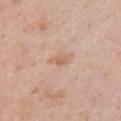Q: Is there a histopathology result?
A: total-body-photography surveillance lesion; no biopsy
Q: Automated lesion metrics?
A: a lesion area of about 3 mm², an eccentricity of roughly 0.85, and a symmetry-axis asymmetry near 0.35; a mean CIELAB color near L≈63 a*≈20 b*≈33, roughly 7 lightness units darker than nearby skin, and a normalized border contrast of about 6.5; a border-irregularity index near 4/10 and internal color variation of about 1 on a 0–10 scale; an automated nevus-likeness rating near 0 out of 100 and a lesion-detection confidence of about 100/100
Q: Illumination type?
A: white-light illumination
Q: What are the patient's age and sex?
A: female, aged 38 to 42
Q: What kind of image is this?
A: ~15 mm crop, total-body skin-cancer survey
Q: Lesion location?
A: the chest
Q: What is the lesion's diameter?
A: about 2.5 mm